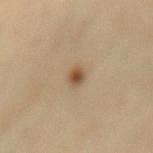{
  "biopsy_status": "not biopsied; imaged during a skin examination",
  "lighting": "cross-polarized",
  "patient": {
    "sex": "male",
    "age_approx": 65
  },
  "lesion_size": {
    "long_diameter_mm_approx": 2.5
  },
  "site": "lower back",
  "automated_metrics": {
    "area_mm2_approx": 3.5,
    "shape_asymmetry": 0.2,
    "border_irregularity_0_10": 2.0,
    "color_variation_0_10": 5.5,
    "lesion_detection_confidence_0_100": 100
  },
  "image": {
    "source": "total-body photography crop",
    "field_of_view_mm": 15
  }
}